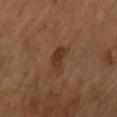Case summary:
– workup — total-body-photography surveillance lesion; no biopsy
– tile lighting — cross-polarized illumination
– TBP lesion metrics — an outline eccentricity of about 0.85 (0 = round, 1 = elongated) and two-axis asymmetry of about 0.25; an automated nevus-likeness rating near 50 out of 100 and a lesion-detection confidence of about 100/100
– lesion size — ~3.5 mm (longest diameter)
– location — the chest
– imaging modality — 15 mm crop, total-body photography